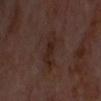follow-up: total-body-photography surveillance lesion; no biopsy
anatomic site: the head or neck
acquisition: 15 mm crop, total-body photography
subject: in their mid- to late 60s
size: about 4 mm